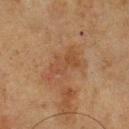| feature | finding |
|---|---|
| follow-up | no biopsy performed (imaged during a skin exam) |
| patient | male, approximately 75 years of age |
| body site | the chest |
| tile lighting | cross-polarized |
| imaging modality | ~15 mm crop, total-body skin-cancer survey |